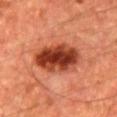The lesion was photographed on a routine skin check and not biopsied; there is no pathology result. A male subject in their mid-60s. Cropped from a total-body skin-imaging series; the visible field is about 15 mm. From the front of the torso.